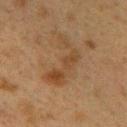Findings:
• notes: imaged on a skin check; not biopsied
• tile lighting: cross-polarized illumination
• image source: total-body-photography crop, ~15 mm field of view
• location: the upper back
• patient: female, aged approximately 40
• automated metrics: an average lesion color of about L≈37 a*≈16 b*≈29 (CIELAB); a within-lesion color-variation index near 4/10 and radial color variation of about 1